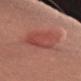Clinical impression:
Captured during whole-body skin photography for melanoma surveillance; the lesion was not biopsied.
Acquisition and patient details:
This image is a 15 mm lesion crop taken from a total-body photograph. On the left thigh. This is a white-light tile. The subject is a female about 65 years old.